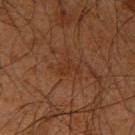{"biopsy_status": "not biopsied; imaged during a skin examination", "patient": {"sex": "male", "age_approx": 65}, "site": "right upper arm", "image": {"source": "total-body photography crop", "field_of_view_mm": 15}}